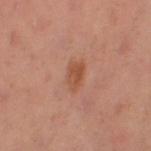Imaged during a routine full-body skin examination; the lesion was not biopsied and no histopathology is available.
A lesion tile, about 15 mm wide, cut from a 3D total-body photograph.
The lesion is located on the left thigh.
A female subject, in their 40s.
The tile uses cross-polarized illumination.
Automated image analysis of the tile measured a footprint of about 4.5 mm², an outline eccentricity of about 0.85 (0 = round, 1 = elongated), and a symmetry-axis asymmetry near 0.25. And it measured a lesion color around L≈50 a*≈24 b*≈33 in CIELAB and a lesion–skin lightness drop of about 8. The analysis additionally found internal color variation of about 3 on a 0–10 scale and peripheral color asymmetry of about 1. It also reported a nevus-likeness score of about 30/100 and a detector confidence of about 100 out of 100 that the crop contains a lesion.